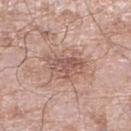  biopsy_status: not biopsied; imaged during a skin examination
  site: right lower leg
  patient:
    sex: male
    age_approx: 60
  lighting: white-light
  lesion_size:
    long_diameter_mm_approx: 4.5
  image:
    source: total-body photography crop
    field_of_view_mm: 15